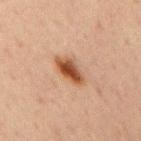Clinical impression:
Imaged during a routine full-body skin examination; the lesion was not biopsied and no histopathology is available.
Acquisition and patient details:
The lesion is on the chest. The recorded lesion diameter is about 4 mm. The patient is a male roughly 70 years of age. Captured under cross-polarized illumination. Cropped from a whole-body photographic skin survey; the tile spans about 15 mm.